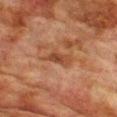<lesion>
<biopsy_status>not biopsied; imaged during a skin examination</biopsy_status>
<patient>
  <sex>male</sex>
  <age_approx>75</age_approx>
</patient>
<lighting>cross-polarized</lighting>
<site>chest</site>
<automated_metrics>
  <area_mm2_approx>5.5</area_mm2_approx>
  <eccentricity>0.85</eccentricity>
  <shape_asymmetry>0.3</shape_asymmetry>
  <cielab_L>38</cielab_L>
  <cielab_a>21</cielab_a>
  <cielab_b>29</cielab_b>
  <vs_skin_darker_L>8.0</vs_skin_darker_L>
  <vs_skin_contrast_norm>7.0</vs_skin_contrast_norm>
  <border_irregularity_0_10>3.5</border_irregularity_0_10>
  <color_variation_0_10>3.0</color_variation_0_10>
  <peripheral_color_asymmetry>1.0</peripheral_color_asymmetry>
</automated_metrics>
<lesion_size>
  <long_diameter_mm_approx>3.5</long_diameter_mm_approx>
</lesion_size>
<image>
  <source>total-body photography crop</source>
  <field_of_view_mm>15</field_of_view_mm>
</image>
</lesion>